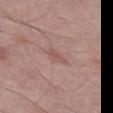Captured under white-light illumination. A 15 mm crop from a total-body photograph taken for skin-cancer surveillance. On the left thigh. A male patient aged around 55.